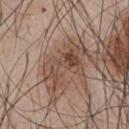Impression: The lesion was tiled from a total-body skin photograph and was not biopsied. Acquisition and patient details: About 5 mm across. The lesion is located on the chest. The subject is a male in their mid-40s. A region of skin cropped from a whole-body photographic capture, roughly 15 mm wide. Automated image analysis of the tile measured a lesion color around L≈46 a*≈18 b*≈25 in CIELAB, a lesion–skin lightness drop of about 9, and a normalized lesion–skin contrast near 7.